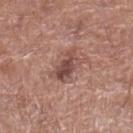Case summary:
* workup · total-body-photography surveillance lesion; no biopsy
* subject · male, in their mid- to late 60s
* diameter · ~4.5 mm (longest diameter)
* acquisition · ~15 mm crop, total-body skin-cancer survey
* illumination · white-light illumination
* location · the left lower leg